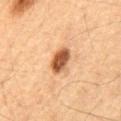Part of a total-body skin-imaging series; this lesion was reviewed on a skin check and was not flagged for biopsy.
On the abdomen.
A male subject roughly 65 years of age.
Captured under cross-polarized illumination.
Cropped from a total-body skin-imaging series; the visible field is about 15 mm.
The total-body-photography lesion software estimated a footprint of about 6.5 mm², an eccentricity of roughly 0.8, and a symmetry-axis asymmetry near 0.15.